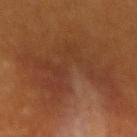Assessment:
This lesion was catalogued during total-body skin photography and was not selected for biopsy.
Acquisition and patient details:
A roughly 15 mm field-of-view crop from a total-body skin photograph. From the lower back. A male subject, about 60 years old.